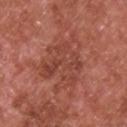Cropped from a total-body skin-imaging series; the visible field is about 15 mm. Imaged with white-light lighting. A male subject, aged 63 to 67. From the upper back. Approximately 7 mm at its widest.